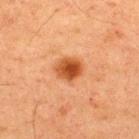Automated tile analysis of the lesion measured a lesion area of about 7 mm², an outline eccentricity of about 0.55 (0 = round, 1 = elongated), and a symmetry-axis asymmetry near 0.15. And it measured a border-irregularity rating of about 1.5/10 and peripheral color asymmetry of about 1.5. It also reported a classifier nevus-likeness of about 100/100.
On the back.
A 15 mm close-up tile from a total-body photography series done for melanoma screening.
Longest diameter approximately 3 mm.
A male subject, aged around 60.